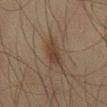Clinical impression: The lesion was photographed on a routine skin check and not biopsied; there is no pathology result. Acquisition and patient details: Approximately 4.5 mm at its widest. A 15 mm close-up extracted from a 3D total-body photography capture. The lesion is located on the right thigh. The lesion-visualizer software estimated a footprint of about 7.5 mm² and a symmetry-axis asymmetry near 0.2. The analysis additionally found an automated nevus-likeness rating near 75 out of 100. The patient is a male aged 43–47. The tile uses cross-polarized illumination.